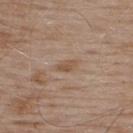Captured during whole-body skin photography for melanoma surveillance; the lesion was not biopsied.
The lesion's longest dimension is about 2.5 mm.
A roughly 15 mm field-of-view crop from a total-body skin photograph.
This is a white-light tile.
On the upper back.
The patient is a male approximately 55 years of age.